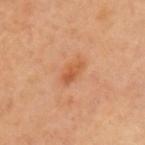notes: catalogued during a skin exam; not biopsied | body site: the left upper arm | illumination: cross-polarized | patient: female, aged 43–47 | acquisition: total-body-photography crop, ~15 mm field of view | TBP lesion metrics: a lesion area of about 5 mm², a shape eccentricity near 0.85, and a symmetry-axis asymmetry near 0.2; a mean CIELAB color near L≈56 a*≈26 b*≈39, roughly 8 lightness units darker than nearby skin, and a lesion-to-skin contrast of about 6.5 (normalized; higher = more distinct); a border-irregularity rating of about 2.5/10 and a peripheral color-asymmetry measure near 1.5; a nevus-likeness score of about 10/100 and a lesion-detection confidence of about 100/100 | lesion diameter: ≈3.5 mm.